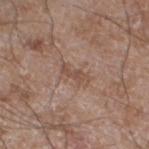Q: Is there a histopathology result?
A: imaged on a skin check; not biopsied
Q: How large is the lesion?
A: ≈3 mm
Q: What lighting was used for the tile?
A: white-light
Q: How was this image acquired?
A: ~15 mm crop, total-body skin-cancer survey
Q: Who is the patient?
A: male, in their 60s
Q: What is the anatomic site?
A: the leg